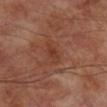| key | value |
|---|---|
| workup | catalogued during a skin exam; not biopsied |
| body site | the leg |
| subject | male, aged around 70 |
| imaging modality | ~15 mm crop, total-body skin-cancer survey |